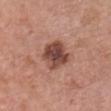| key | value |
|---|---|
| follow-up | imaged on a skin check; not biopsied |
| automated lesion analysis | an area of roughly 11 mm², an eccentricity of roughly 0.4, and a shape-asymmetry score of about 0.2 (0 = symmetric); an average lesion color of about L≈46 a*≈23 b*≈26 (CIELAB), roughly 14 lightness units darker than nearby skin, and a lesion-to-skin contrast of about 10 (normalized; higher = more distinct); a color-variation rating of about 5.5/10 and peripheral color asymmetry of about 1.5; a classifier nevus-likeness of about 10/100 and lesion-presence confidence of about 100/100 |
| body site | the chest |
| image | total-body-photography crop, ~15 mm field of view |
| lesion size | ~4 mm (longest diameter) |
| lighting | white-light illumination |
| subject | female, about 60 years old |